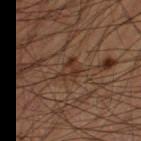{"biopsy_status": "not biopsied; imaged during a skin examination", "patient": {"sex": "male", "age_approx": 60}, "image": {"source": "total-body photography crop", "field_of_view_mm": 15}, "site": "left thigh"}